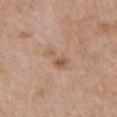Imaged during a routine full-body skin examination; the lesion was not biopsied and no histopathology is available. A 15 mm close-up tile from a total-body photography series done for melanoma screening. Imaged with white-light lighting. From the chest. The lesion's longest dimension is about 4.5 mm. A female patient aged 38 to 42. Automated tile analysis of the lesion measured a lesion area of about 8.5 mm², an eccentricity of roughly 0.85, and a shape-asymmetry score of about 0.3 (0 = symmetric). And it measured about 6 CIELAB-L* units darker than the surrounding skin and a lesion-to-skin contrast of about 5 (normalized; higher = more distinct). The software also gave a within-lesion color-variation index near 5.5/10 and peripheral color asymmetry of about 1.5.